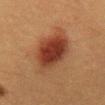Impression:
The lesion was tiled from a total-body skin photograph and was not biopsied.
Acquisition and patient details:
A roughly 15 mm field-of-view crop from a total-body skin photograph. A female patient roughly 40 years of age. From the abdomen.